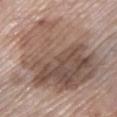workup = total-body-photography surveillance lesion; no biopsy